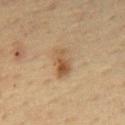A male subject about 65 years old. Approximately 3.5 mm at its widest. Located on the mid back. A 15 mm crop from a total-body photograph taken for skin-cancer surveillance. Imaged with cross-polarized lighting.